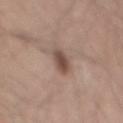Background:
A male subject roughly 65 years of age. The total-body-photography lesion software estimated a mean CIELAB color near L≈48 a*≈17 b*≈24, a lesion–skin lightness drop of about 12, and a normalized lesion–skin contrast near 8.5. And it measured a border-irregularity rating of about 1.5/10, a color-variation rating of about 2.5/10, and a peripheral color-asymmetry measure near 1. This is a white-light tile. The lesion is located on the mid back. A roughly 15 mm field-of-view crop from a total-body skin photograph.